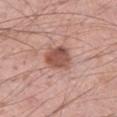<lesion>
<biopsy_status>not biopsied; imaged during a skin examination</biopsy_status>
<image>
  <source>total-body photography crop</source>
  <field_of_view_mm>15</field_of_view_mm>
</image>
<site>left thigh</site>
<patient>
  <sex>male</sex>
  <age_approx>55</age_approx>
</patient>
</lesion>